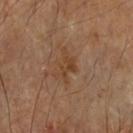Part of a total-body skin-imaging series; this lesion was reviewed on a skin check and was not flagged for biopsy. This image is a 15 mm lesion crop taken from a total-body photograph. This is a cross-polarized tile. Automated image analysis of the tile measured a lesion area of about 6 mm², an eccentricity of roughly 0.8, and a symmetry-axis asymmetry near 0.4. The software also gave about 6 CIELAB-L* units darker than the surrounding skin and a lesion-to-skin contrast of about 6 (normalized; higher = more distinct). The software also gave a border-irregularity index near 6/10, a color-variation rating of about 2.5/10, and peripheral color asymmetry of about 1. The software also gave a classifier nevus-likeness of about 0/100. About 4 mm across. Located on the left forearm.